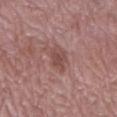patient: female, approximately 70 years of age | automated metrics: a lesion color around L≈48 a*≈21 b*≈22 in CIELAB, a lesion–skin lightness drop of about 8, and a lesion-to-skin contrast of about 6.5 (normalized; higher = more distinct) | image: ~15 mm crop, total-body skin-cancer survey | site: the leg | lighting: white-light illumination.